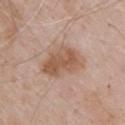This lesion was catalogued during total-body skin photography and was not selected for biopsy. This image is a 15 mm lesion crop taken from a total-body photograph. A male subject, roughly 70 years of age. The lesion's longest dimension is about 6 mm. The lesion is on the head or neck.